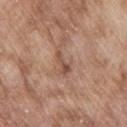notes = imaged on a skin check; not biopsied.